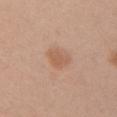notes = imaged on a skin check; not biopsied
diameter = ≈3 mm
image source = ~15 mm crop, total-body skin-cancer survey
patient = female, aged approximately 35
site = the arm
lighting = white-light
image-analysis metrics = a border-irregularity index near 2.5/10, a within-lesion color-variation index near 1.5/10, and radial color variation of about 0.5; an automated nevus-likeness rating near 15 out of 100